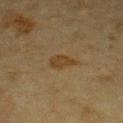biopsy status — no biopsy performed (imaged during a skin exam)
lesion size — about 3.5 mm
body site — the chest
image source — total-body-photography crop, ~15 mm field of view
subject — male, about 85 years old or older
lighting — cross-polarized illumination